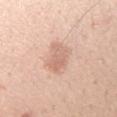follow-up=imaged on a skin check; not biopsied | image=total-body-photography crop, ~15 mm field of view | TBP lesion metrics=a footprint of about 7 mm² and a symmetry-axis asymmetry near 0.35; a border-irregularity index near 3.5/10, a color-variation rating of about 2.5/10, and radial color variation of about 1; a detector confidence of about 100 out of 100 that the crop contains a lesion | patient=female, aged 23 to 27 | tile lighting=white-light illumination | site=the left forearm | diameter=about 3.5 mm.